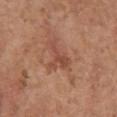| key | value |
|---|---|
| lesion diameter | ~4 mm (longest diameter) |
| subject | female, aged around 65 |
| automated lesion analysis | a footprint of about 5 mm² and a symmetry-axis asymmetry near 0.7; border irregularity of about 9.5 on a 0–10 scale and a color-variation rating of about 0.5/10; an automated nevus-likeness rating near 0 out of 100 and a lesion-detection confidence of about 100/100 |
| location | the left upper arm |
| imaging modality | ~15 mm crop, total-body skin-cancer survey |